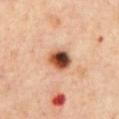An algorithmic analysis of the crop reported a footprint of about 7 mm² and an eccentricity of roughly 0.5. The analysis additionally found an average lesion color of about L≈49 a*≈24 b*≈33 (CIELAB), roughly 22 lightness units darker than nearby skin, and a normalized border contrast of about 14. The analysis additionally found a border-irregularity rating of about 1.5/10, a within-lesion color-variation index near 10/10, and peripheral color asymmetry of about 4.5. It also reported an automated nevus-likeness rating near 100 out of 100. From the chest. A lesion tile, about 15 mm wide, cut from a 3D total-body photograph. The patient is a female aged 43 to 47.